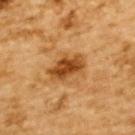Case summary:
– follow-up — total-body-photography surveillance lesion; no biopsy
– patient — male, about 85 years old or older
– diameter — ≈4.5 mm
– body site — the upper back
– tile lighting — cross-polarized
– acquisition — total-body-photography crop, ~15 mm field of view